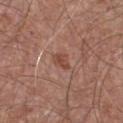| feature | finding |
|---|---|
| follow-up | imaged on a skin check; not biopsied |
| lesion diameter | ~2.5 mm (longest diameter) |
| body site | the chest |
| lighting | white-light illumination |
| image source | ~15 mm tile from a whole-body skin photo |
| patient | male, aged approximately 65 |